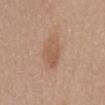Q: Was a biopsy performed?
A: catalogued during a skin exam; not biopsied
Q: What lighting was used for the tile?
A: white-light illumination
Q: Who is the patient?
A: female, about 60 years old
Q: What kind of image is this?
A: 15 mm crop, total-body photography
Q: Where on the body is the lesion?
A: the front of the torso
Q: What is the lesion's diameter?
A: ≈4.5 mm